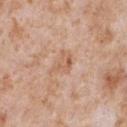Q: Was this lesion biopsied?
A: imaged on a skin check; not biopsied
Q: How was this image acquired?
A: ~15 mm tile from a whole-body skin photo
Q: Who is the patient?
A: male, in their mid- to late 60s
Q: What is the anatomic site?
A: the chest